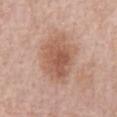Q: Was this lesion biopsied?
A: imaged on a skin check; not biopsied
Q: What is the lesion's diameter?
A: ≈6.5 mm
Q: What is the imaging modality?
A: 15 mm crop, total-body photography
Q: What are the patient's age and sex?
A: female, approximately 75 years of age
Q: Automated lesion metrics?
A: an area of roughly 21 mm², an eccentricity of roughly 0.7, and two-axis asymmetry of about 0.15; roughly 10 lightness units darker than nearby skin and a lesion-to-skin contrast of about 7.5 (normalized; higher = more distinct); a border-irregularity rating of about 2/10 and radial color variation of about 1.5; a classifier nevus-likeness of about 70/100 and a detector confidence of about 100 out of 100 that the crop contains a lesion
Q: How was the tile lit?
A: white-light
Q: Lesion location?
A: the abdomen